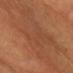No biopsy was performed on this lesion — it was imaged during a full skin examination and was not determined to be concerning.